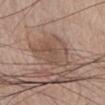follow-up = no biopsy performed (imaged during a skin exam)
illumination = white-light illumination
size = ~5 mm (longest diameter)
subject = male, aged 68–72
acquisition = ~15 mm crop, total-body skin-cancer survey
site = the chest
image-analysis metrics = a border-irregularity rating of about 3/10 and peripheral color asymmetry of about 1.5; a nevus-likeness score of about 15/100 and a lesion-detection confidence of about 95/100